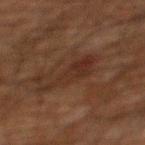Imaged during a routine full-body skin examination; the lesion was not biopsied and no histopathology is available.
A male subject, aged 58 to 62.
The lesion is located on the back.
Approximately 6 mm at its widest.
The tile uses cross-polarized illumination.
A close-up tile cropped from a whole-body skin photograph, about 15 mm across.
Automated image analysis of the tile measured an average lesion color of about L≈22 a*≈15 b*≈20 (CIELAB), a lesion–skin lightness drop of about 5, and a normalized border contrast of about 6. It also reported a nevus-likeness score of about 0/100 and a detector confidence of about 100 out of 100 that the crop contains a lesion.